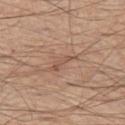notes: total-body-photography surveillance lesion; no biopsy
image: ~15 mm crop, total-body skin-cancer survey
subject: male, aged 63–67
lesion size: ~3.5 mm (longest diameter)
anatomic site: the leg
tile lighting: white-light